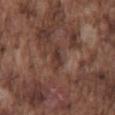Case summary:
* automated metrics — about 7 CIELAB-L* units darker than the surrounding skin; a border-irregularity index near 3.5/10 and radial color variation of about 0.5; a nevus-likeness score of about 0/100 and a lesion-detection confidence of about 60/100
* illumination — white-light
* anatomic site — the mid back
* lesion size — ≈3 mm
* imaging modality — ~15 mm crop, total-body skin-cancer survey
* patient — male, about 75 years old